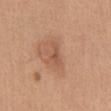Findings:
– notes: total-body-photography surveillance lesion; no biopsy
– diameter: ≈3 mm
– image: total-body-photography crop, ~15 mm field of view
– subject: female, in their 40s
– illumination: white-light illumination
– site: the chest